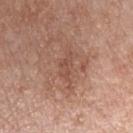Acquisition and patient details: A male subject aged approximately 50. About 3.5 mm across. A close-up tile cropped from a whole-body skin photograph, about 15 mm across. Located on the chest. The tile uses white-light illumination. The total-body-photography lesion software estimated a footprint of about 3 mm² and a shape-asymmetry score of about 0.6 (0 = symmetric). The analysis additionally found roughly 7 lightness units darker than nearby skin and a lesion-to-skin contrast of about 5 (normalized; higher = more distinct). The analysis additionally found border irregularity of about 8.5 on a 0–10 scale, internal color variation of about 0 on a 0–10 scale, and a peripheral color-asymmetry measure near 0. And it measured an automated nevus-likeness rating near 0 out of 100 and a detector confidence of about 95 out of 100 that the crop contains a lesion.